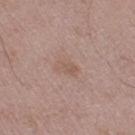Assessment:
Part of a total-body skin-imaging series; this lesion was reviewed on a skin check and was not flagged for biopsy.
Acquisition and patient details:
A male subject about 50 years old. This image is a 15 mm lesion crop taken from a total-body photograph. From the left thigh. Automated tile analysis of the lesion measured a mean CIELAB color near L≈56 a*≈17 b*≈25 and a normalized border contrast of about 4.5. It also reported a nevus-likeness score of about 0/100. The tile uses white-light illumination.